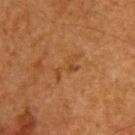Clinical impression:
The lesion was photographed on a routine skin check and not biopsied; there is no pathology result.
Image and clinical context:
A 15 mm close-up tile from a total-body photography series done for melanoma screening. From the upper back. A male patient, aged around 65.